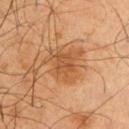The lesion was tiled from a total-body skin photograph and was not biopsied.
The tile uses cross-polarized illumination.
An algorithmic analysis of the crop reported a lesion area of about 12 mm², a shape eccentricity near 0.6, and a symmetry-axis asymmetry near 0.35. And it measured a peripheral color-asymmetry measure near 1.
A lesion tile, about 15 mm wide, cut from a 3D total-body photograph.
A male patient, aged around 65.
Located on the left upper arm.
Longest diameter approximately 5 mm.